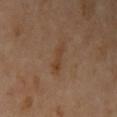  patient:
    sex: female
    age_approx: 65
  lesion_size:
    long_diameter_mm_approx: 3.5
  site: left upper arm
  lighting: cross-polarized
  image:
    source: total-body photography crop
    field_of_view_mm: 15